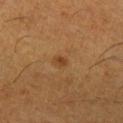notes: catalogued during a skin exam; not biopsied
diameter: ~1.5 mm (longest diameter)
subject: male, approximately 60 years of age
image: ~15 mm tile from a whole-body skin photo
lighting: cross-polarized illumination
location: the right lower leg